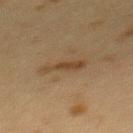Recorded during total-body skin imaging; not selected for excision or biopsy.